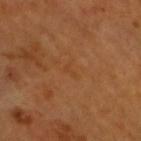Imaged during a routine full-body skin examination; the lesion was not biopsied and no histopathology is available.
Longest diameter approximately 2 mm.
The lesion is located on the head or neck.
Captured under cross-polarized illumination.
The patient is a male in their 60s.
A 15 mm close-up extracted from a 3D total-body photography capture.